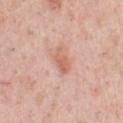No biopsy was performed on this lesion — it was imaged during a full skin examination and was not determined to be concerning.
This is a white-light tile.
The subject is a male aged around 40.
Located on the chest.
A close-up tile cropped from a whole-body skin photograph, about 15 mm across.
The lesion-visualizer software estimated a footprint of about 4.5 mm² and an eccentricity of roughly 0.85. It also reported a mean CIELAB color near L≈63 a*≈24 b*≈31, roughly 9 lightness units darker than nearby skin, and a normalized border contrast of about 6.5.